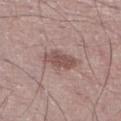Findings:
* workup — no biopsy performed (imaged during a skin exam)
* image source — ~15 mm tile from a whole-body skin photo
* body site — the right lower leg
* diameter — ~4 mm (longest diameter)
* lighting — white-light illumination
* subject — male, about 35 years old